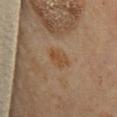The lesion was tiled from a total-body skin photograph and was not biopsied.
A female patient, aged 63–67.
On the mid back.
This image is a 15 mm lesion crop taken from a total-body photograph.
Longest diameter approximately 3 mm.
Captured under cross-polarized illumination.
The lesion-visualizer software estimated an area of roughly 4.5 mm², an eccentricity of roughly 0.85, and two-axis asymmetry of about 0.3. The analysis additionally found a mean CIELAB color near L≈46 a*≈18 b*≈33, a lesion–skin lightness drop of about 7, and a normalized border contrast of about 7. The analysis additionally found a border-irregularity rating of about 3/10 and a color-variation rating of about 1/10.